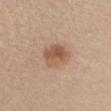Clinical impression:
Captured during whole-body skin photography for melanoma surveillance; the lesion was not biopsied.
Background:
The lesion is located on the chest. A female subject, in their 30s. A 15 mm crop from a total-body photograph taken for skin-cancer surveillance.